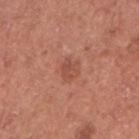{"lighting": "white-light", "patient": {"sex": "male", "age_approx": 45}, "site": "left upper arm", "lesion_size": {"long_diameter_mm_approx": 2.5}, "image": {"source": "total-body photography crop", "field_of_view_mm": 15}}